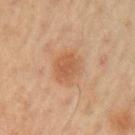| field | value |
|---|---|
| illumination | cross-polarized illumination |
| automated metrics | a lesion area of about 9.5 mm²; a within-lesion color-variation index near 2.5/10 and radial color variation of about 1; an automated nevus-likeness rating near 55 out of 100 |
| site | the left upper arm |
| lesion diameter | ~4 mm (longest diameter) |
| image source | total-body-photography crop, ~15 mm field of view |
| subject | male, in their 60s |